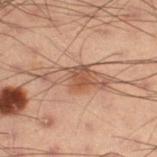Recorded during total-body skin imaging; not selected for excision or biopsy.
The tile uses cross-polarized illumination.
From the left thigh.
A male subject, aged 53 to 57.
A 15 mm close-up tile from a total-body photography series done for melanoma screening.